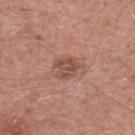Notes:
• notes · total-body-photography surveillance lesion; no biopsy
• imaging modality · total-body-photography crop, ~15 mm field of view
• illumination · white-light illumination
• location · the mid back
• subject · male, aged approximately 55
• lesion diameter · ≈3.5 mm